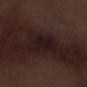No biopsy was performed on this lesion — it was imaged during a full skin examination and was not determined to be concerning. The lesion is located on the right lower leg. The patient is a male aged around 70. Automated tile analysis of the lesion measured a lesion-to-skin contrast of about 9 (normalized; higher = more distinct). A roughly 15 mm field-of-view crop from a total-body skin photograph. About 3 mm across. This is a white-light tile.